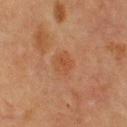Case summary:
* biopsy status — catalogued during a skin exam; not biopsied
* location — the upper back
* subject — male, approximately 60 years of age
* tile lighting — cross-polarized
* image source — ~15 mm crop, total-body skin-cancer survey